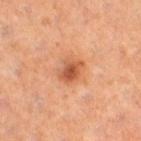Assessment:
No biopsy was performed on this lesion — it was imaged during a full skin examination and was not determined to be concerning.